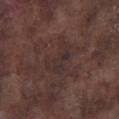Clinical impression: No biopsy was performed on this lesion — it was imaged during a full skin examination and was not determined to be concerning. Background: Located on the left lower leg. Automated image analysis of the tile measured a lesion color around L≈29 a*≈15 b*≈16 in CIELAB. The subject is a male in their mid-70s. Captured under white-light illumination. The lesion's longest dimension is about 4.5 mm. Cropped from a whole-body photographic skin survey; the tile spans about 15 mm.